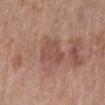Impression:
The lesion was photographed on a routine skin check and not biopsied; there is no pathology result.
Clinical summary:
The lesion is on the left lower leg. Cropped from a total-body skin-imaging series; the visible field is about 15 mm. The patient is a female aged approximately 65.